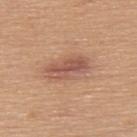Impression:
The lesion was photographed on a routine skin check and not biopsied; there is no pathology result.
Context:
A 15 mm close-up tile from a total-body photography series done for melanoma screening. Located on the upper back. Measured at roughly 5 mm in maximum diameter. The subject is a female aged approximately 60. Captured under white-light illumination.